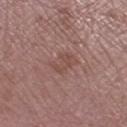| feature | finding |
|---|---|
| biopsy status | no biopsy performed (imaged during a skin exam) |
| automated metrics | a classifier nevus-likeness of about 0/100 and lesion-presence confidence of about 100/100 |
| subject | female, aged 68–72 |
| lesion diameter | ≈3 mm |
| anatomic site | the left thigh |
| tile lighting | white-light |
| imaging modality | ~15 mm tile from a whole-body skin photo |